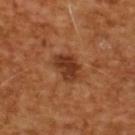Cropped from a whole-body photographic skin survey; the tile spans about 15 mm. This is a cross-polarized tile. A male subject about 65 years old.